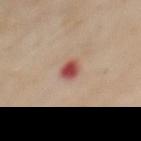workup = total-body-photography surveillance lesion; no biopsy
subject = male, about 70 years old
image-analysis metrics = an area of roughly 4 mm², an eccentricity of roughly 0.65, and two-axis asymmetry of about 0.2
location = the front of the torso
acquisition = ~15 mm tile from a whole-body skin photo
lighting = cross-polarized